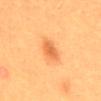The lesion was tiled from a total-body skin photograph and was not biopsied. Captured under cross-polarized illumination. About 3.5 mm across. A female subject aged approximately 30. Automated image analysis of the tile measured a footprint of about 5.5 mm², a shape eccentricity near 0.85, and a shape-asymmetry score of about 0.15 (0 = symmetric). And it measured a lesion color around L≈62 a*≈29 b*≈45 in CIELAB, a lesion–skin lightness drop of about 11, and a normalized lesion–skin contrast near 6.5. The software also gave an automated nevus-likeness rating near 95 out of 100. A 15 mm crop from a total-body photograph taken for skin-cancer surveillance. The lesion is on the mid back.